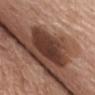Recorded during total-body skin imaging; not selected for excision or biopsy.
The lesion's longest dimension is about 5.5 mm.
Imaged with white-light lighting.
From the left thigh.
The patient is a female in their mid- to late 70s.
A lesion tile, about 15 mm wide, cut from a 3D total-body photograph.